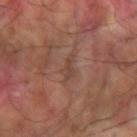Q: Was a biopsy performed?
A: catalogued during a skin exam; not biopsied
Q: Lesion size?
A: about 2.5 mm
Q: Who is the patient?
A: male, in their 60s
Q: What lighting was used for the tile?
A: cross-polarized illumination
Q: Lesion location?
A: the left forearm
Q: What is the imaging modality?
A: ~15 mm tile from a whole-body skin photo